Cropped from a whole-body photographic skin survey; the tile spans about 15 mm. Automated tile analysis of the lesion measured roughly 18 lightness units darker than nearby skin and a normalized lesion–skin contrast near 13.5. From the right thigh. A female subject approximately 65 years of age. Measured at roughly 2 mm in maximum diameter — 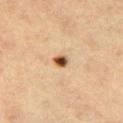Histopathological examination showed a benign lesion: dysplastic (Clark) nevus.